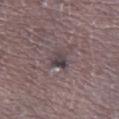Q: Was this lesion biopsied?
A: imaged on a skin check; not biopsied
Q: Patient demographics?
A: male, aged 48 to 52
Q: What is the imaging modality?
A: ~15 mm crop, total-body skin-cancer survey
Q: What is the anatomic site?
A: the left lower leg
Q: How was the tile lit?
A: white-light
Q: How large is the lesion?
A: ~2.5 mm (longest diameter)